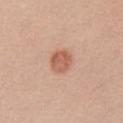| key | value |
|---|---|
| biopsy status | catalogued during a skin exam; not biopsied |
| patient | female, aged 23–27 |
| anatomic site | the chest |
| imaging modality | 15 mm crop, total-body photography |
| automated lesion analysis | an automated nevus-likeness rating near 95 out of 100 |